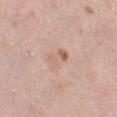A 15 mm close-up tile from a total-body photography series done for melanoma screening.
The patient is a female aged 38 to 42.
Located on the left lower leg.